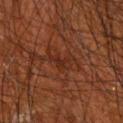<case>
  <biopsy_status>not biopsied; imaged during a skin examination</biopsy_status>
</case>